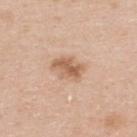Notes:
• workup — no biopsy performed (imaged during a skin exam)